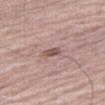Clinical impression: This lesion was catalogued during total-body skin photography and was not selected for biopsy. Background: A region of skin cropped from a whole-body photographic capture, roughly 15 mm wide. Located on the right thigh. A male patient roughly 80 years of age.